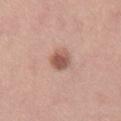The lesion was photographed on a routine skin check and not biopsied; there is no pathology result.
A female subject in their mid-20s.
Longest diameter approximately 3 mm.
Located on the right thigh.
Imaged with white-light lighting.
Automated image analysis of the tile measured internal color variation of about 3.5 on a 0–10 scale and peripheral color asymmetry of about 1. It also reported a nevus-likeness score of about 95/100 and lesion-presence confidence of about 100/100.
A roughly 15 mm field-of-view crop from a total-body skin photograph.